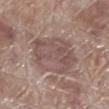This lesion was catalogued during total-body skin photography and was not selected for biopsy.
The lesion is on the left lower leg.
A lesion tile, about 15 mm wide, cut from a 3D total-body photograph.
The subject is a male roughly 70 years of age.